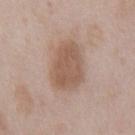Q: Was this lesion biopsied?
A: no biopsy performed (imaged during a skin exam)
Q: How was this image acquired?
A: ~15 mm tile from a whole-body skin photo
Q: What is the lesion's diameter?
A: about 5.5 mm
Q: What are the patient's age and sex?
A: male, about 50 years old
Q: How was the tile lit?
A: white-light
Q: What is the anatomic site?
A: the chest